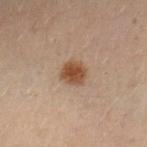Captured during whole-body skin photography for melanoma surveillance; the lesion was not biopsied. The total-body-photography lesion software estimated a lesion area of about 6.5 mm², a shape eccentricity near 0.3, and a symmetry-axis asymmetry near 0.2. And it measured about 10 CIELAB-L* units darker than the surrounding skin and a normalized lesion–skin contrast near 9.5. The analysis additionally found a border-irregularity rating of about 1.5/10 and a color-variation rating of about 2.5/10. Imaged with cross-polarized lighting. A female patient, aged 28–32. From the left forearm. A 15 mm close-up extracted from a 3D total-body photography capture. The recorded lesion diameter is about 3 mm.